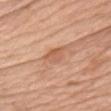biopsy status=imaged on a skin check; not biopsied